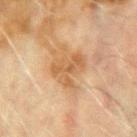No biopsy was performed on this lesion — it was imaged during a full skin examination and was not determined to be concerning. Captured under cross-polarized illumination. An algorithmic analysis of the crop reported an average lesion color of about L≈49 a*≈18 b*≈33 (CIELAB). And it measured a border-irregularity rating of about 6/10, a within-lesion color-variation index near 3.5/10, and a peripheral color-asymmetry measure near 1. A male subject, approximately 70 years of age. A 15 mm crop from a total-body photograph taken for skin-cancer surveillance. About 4.5 mm across. From the left upper arm.